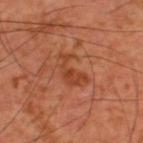{"biopsy_status": "not biopsied; imaged during a skin examination"}